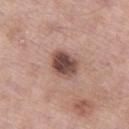Case summary:
• diameter · about 3.5 mm
• automated metrics · a footprint of about 8.5 mm² and an outline eccentricity of about 0.6 (0 = round, 1 = elongated)
• acquisition · total-body-photography crop, ~15 mm field of view
• subject · male, roughly 55 years of age
• lighting · white-light illumination
• location · the left thigh